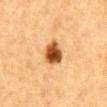No biopsy was performed on this lesion — it was imaged during a full skin examination and was not determined to be concerning. Located on the abdomen. A male subject, aged 83 or over. Captured under cross-polarized illumination. A 15 mm close-up tile from a total-body photography series done for melanoma screening. The lesion-visualizer software estimated a lesion area of about 7.5 mm² and an outline eccentricity of about 0.55 (0 = round, 1 = elongated). And it measured a border-irregularity rating of about 2/10, a color-variation rating of about 6.5/10, and radial color variation of about 2.